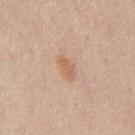Part of a total-body skin-imaging series; this lesion was reviewed on a skin check and was not flagged for biopsy. Located on the mid back. A male subject, approximately 60 years of age. A 15 mm close-up tile from a total-body photography series done for melanoma screening. About 2.5 mm across.